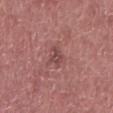Q: Was this lesion biopsied?
A: total-body-photography surveillance lesion; no biopsy
Q: What is the lesion's diameter?
A: ~2.5 mm (longest diameter)
Q: Illumination type?
A: white-light illumination
Q: What is the imaging modality?
A: ~15 mm crop, total-body skin-cancer survey
Q: Where on the body is the lesion?
A: the back
Q: Patient demographics?
A: male, roughly 60 years of age